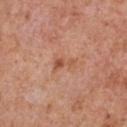Findings:
- workup · total-body-photography surveillance lesion; no biopsy
- acquisition · ~15 mm crop, total-body skin-cancer survey
- anatomic site · the front of the torso
- subject · male, roughly 65 years of age
- lesion diameter · about 3 mm
- lighting · white-light illumination